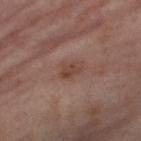Q: Was this lesion biopsied?
A: no biopsy performed (imaged during a skin exam)
Q: What are the patient's age and sex?
A: female, in their mid- to late 50s
Q: Lesion location?
A: the right thigh
Q: Automated lesion metrics?
A: a border-irregularity rating of about 3/10 and radial color variation of about 1
Q: How large is the lesion?
A: about 3 mm
Q: What kind of image is this?
A: ~15 mm tile from a whole-body skin photo
Q: How was the tile lit?
A: cross-polarized